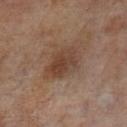Recorded during total-body skin imaging; not selected for excision or biopsy. The lesion's longest dimension is about 5 mm. From the left lower leg. A male subject, aged 68 to 72. A 15 mm close-up extracted from a 3D total-body photography capture. The tile uses cross-polarized illumination.